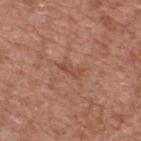Case summary:
• workup: no biopsy performed (imaged during a skin exam)
• image-analysis metrics: a border-irregularity index near 5.5/10 and a peripheral color-asymmetry measure near 0
• patient: male, aged approximately 65
• anatomic site: the upper back
• tile lighting: white-light
• image: ~15 mm tile from a whole-body skin photo